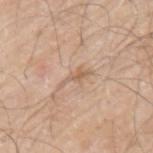notes: catalogued during a skin exam; not biopsied | diameter: ~3.5 mm (longest diameter) | subject: male, aged approximately 65 | image-analysis metrics: an area of roughly 3 mm², an outline eccentricity of about 0.95 (0 = round, 1 = elongated), and two-axis asymmetry of about 0.65; an average lesion color of about L≈61 a*≈17 b*≈30 (CIELAB), a lesion–skin lightness drop of about 8, and a normalized border contrast of about 5.5; border irregularity of about 8 on a 0–10 scale and internal color variation of about 0 on a 0–10 scale; an automated nevus-likeness rating near 0 out of 100 and a detector confidence of about 85 out of 100 that the crop contains a lesion | image: 15 mm crop, total-body photography | lighting: white-light | site: the left upper arm.